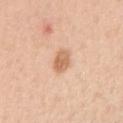{
  "biopsy_status": "not biopsied; imaged during a skin examination",
  "site": "arm",
  "patient": {
    "sex": "female",
    "age_approx": 40
  },
  "lighting": "white-light",
  "image": {
    "source": "total-body photography crop",
    "field_of_view_mm": 15
  },
  "automated_metrics": {
    "area_mm2_approx": 5.0,
    "eccentricity": 0.7,
    "border_irregularity_0_10": 1.5,
    "color_variation_0_10": 3.5
  },
  "lesion_size": {
    "long_diameter_mm_approx": 3.0
  }
}